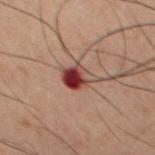notes: catalogued during a skin exam; not biopsied | tile lighting: cross-polarized illumination | patient: male, aged 58–62 | anatomic site: the chest | image source: total-body-photography crop, ~15 mm field of view | lesion size: ≈3 mm | automated metrics: an average lesion color of about L≈31 a*≈21 b*≈20 (CIELAB) and a normalized border contrast of about 11.5; a border-irregularity rating of about 2/10 and a peripheral color-asymmetry measure near 5.